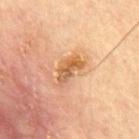No biopsy was performed on this lesion — it was imaged during a full skin examination and was not determined to be concerning. A 15 mm crop from a total-body photograph taken for skin-cancer surveillance. The subject is a male aged approximately 85 or older. Located on the chest. This is a cross-polarized tile.